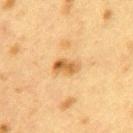Assessment:
Captured during whole-body skin photography for melanoma surveillance; the lesion was not biopsied.
Clinical summary:
A female subject, aged 38 to 42. Cropped from a total-body skin-imaging series; the visible field is about 15 mm. Captured under cross-polarized illumination. The lesion is located on the mid back.